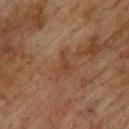biopsy status = no biopsy performed (imaged during a skin exam) | patient = male, roughly 75 years of age | TBP lesion metrics = a border-irregularity index near 6/10, internal color variation of about 2.5 on a 0–10 scale, and radial color variation of about 1; lesion-presence confidence of about 100/100 | diameter = about 3 mm | site = the upper back | tile lighting = cross-polarized | image = ~15 mm tile from a whole-body skin photo.